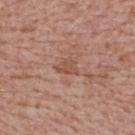The lesion was tiled from a total-body skin photograph and was not biopsied. A 15 mm close-up extracted from a 3D total-body photography capture. About 2.5 mm across. The tile uses white-light illumination. Located on the upper back. A female patient in their mid-60s.